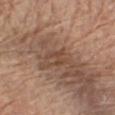Impression: Imaged during a routine full-body skin examination; the lesion was not biopsied and no histopathology is available. Context: Longest diameter approximately 2.5 mm. A male patient, aged approximately 70. The lesion is located on the chest. A roughly 15 mm field-of-view crop from a total-body skin photograph. The tile uses white-light illumination.